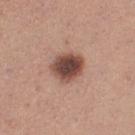Recorded during total-body skin imaging; not selected for excision or biopsy.
Imaged with white-light lighting.
A female patient roughly 30 years of age.
On the left thigh.
A region of skin cropped from a whole-body photographic capture, roughly 15 mm wide.
The lesion's longest dimension is about 4 mm.